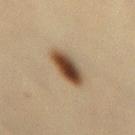  biopsy_status: not biopsied; imaged during a skin examination
  lesion_size:
    long_diameter_mm_approx: 4.0
  patient:
    sex: female
    age_approx: 45
  lighting: cross-polarized
  site: mid back
  automated_metrics:
    area_mm2_approx: 9.5
    eccentricity: 0.75
    shape_asymmetry: 0.1
    nevus_likeness_0_100: 100
    lesion_detection_confidence_0_100: 100
  image:
    source: total-body photography crop
    field_of_view_mm: 15